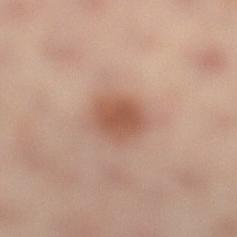| feature | finding |
|---|---|
| notes | total-body-photography surveillance lesion; no biopsy |